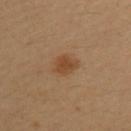{
  "biopsy_status": "not biopsied; imaged during a skin examination",
  "automated_metrics": {
    "border_irregularity_0_10": 2.0,
    "color_variation_0_10": 2.0,
    "lesion_detection_confidence_0_100": 100
  },
  "lesion_size": {
    "long_diameter_mm_approx": 3.0
  },
  "site": "upper back",
  "patient": {
    "sex": "female",
    "age_approx": 35
  },
  "image": {
    "source": "total-body photography crop",
    "field_of_view_mm": 15
  },
  "lighting": "cross-polarized"
}